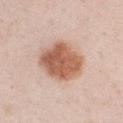Impression:
The lesion was photographed on a routine skin check and not biopsied; there is no pathology result.
Image and clinical context:
The subject is a male in their 50s. Cropped from a whole-body photographic skin survey; the tile spans about 15 mm. The lesion is on the left upper arm.